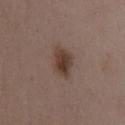automated lesion analysis: an area of roughly 7.5 mm² and two-axis asymmetry of about 0.3; an average lesion color of about L≈39 a*≈15 b*≈23 (CIELAB), a lesion–skin lightness drop of about 10, and a lesion-to-skin contrast of about 9 (normalized; higher = more distinct); a nevus-likeness score of about 100/100 and a detector confidence of about 100 out of 100 that the crop contains a lesion
image source: 15 mm crop, total-body photography
subject: female, about 35 years old
site: the chest